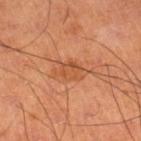{
  "biopsy_status": "not biopsied; imaged during a skin examination",
  "patient": {
    "sex": "male",
    "age_approx": 60
  },
  "site": "right lower leg",
  "automated_metrics": {
    "border_irregularity_0_10": 4.0,
    "color_variation_0_10": 3.0,
    "peripheral_color_asymmetry": 1.0,
    "lesion_detection_confidence_0_100": 100
  },
  "lesion_size": {
    "long_diameter_mm_approx": 4.0
  },
  "image": {
    "source": "total-body photography crop",
    "field_of_view_mm": 15
  },
  "lighting": "cross-polarized"
}